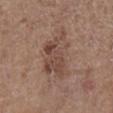biopsy status: catalogued during a skin exam; not biopsied
imaging modality: 15 mm crop, total-body photography
automated lesion analysis: a mean CIELAB color near L≈46 a*≈18 b*≈25, roughly 8 lightness units darker than nearby skin, and a normalized border contrast of about 6.5
subject: female, approximately 65 years of age
lighting: white-light
anatomic site: the right lower leg